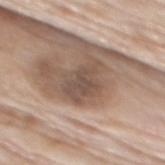{"biopsy_status": "not biopsied; imaged during a skin examination", "lesion_size": {"long_diameter_mm_approx": 4.5}, "automated_metrics": {"cielab_L": 50, "cielab_a": 15, "cielab_b": 24, "vs_skin_darker_L": 10.0, "nevus_likeness_0_100": 0}, "patient": {"sex": "male", "age_approx": "85+"}, "image": {"source": "total-body photography crop", "field_of_view_mm": 15}, "lighting": "white-light", "site": "upper back"}